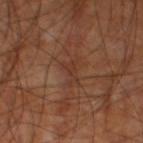No biopsy was performed on this lesion — it was imaged during a full skin examination and was not determined to be concerning. On the left thigh. A male patient aged around 60. Cropped from a whole-body photographic skin survey; the tile spans about 15 mm.